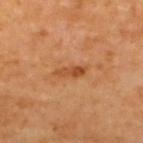{
  "biopsy_status": "not biopsied; imaged during a skin examination",
  "site": "upper back",
  "image": {
    "source": "total-body photography crop",
    "field_of_view_mm": 15
  },
  "automated_metrics": {
    "area_mm2_approx": 4.5,
    "eccentricity": 0.95,
    "shape_asymmetry": 0.25,
    "vs_skin_contrast_norm": 7.0,
    "border_irregularity_0_10": 3.0
  },
  "patient": {
    "age_approx": 65
  },
  "lighting": "cross-polarized"
}